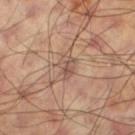Clinical impression:
The lesion was photographed on a routine skin check and not biopsied; there is no pathology result.
Clinical summary:
From the left thigh. The tile uses cross-polarized illumination. The recorded lesion diameter is about 2.5 mm. A 15 mm crop from a total-body photograph taken for skin-cancer surveillance. The patient is a male approximately 60 years of age.